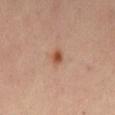workup: total-body-photography surveillance lesion; no biopsy
body site: the abdomen
patient: male, about 65 years old
acquisition: 15 mm crop, total-body photography
lighting: cross-polarized illumination
size: about 2 mm
TBP lesion metrics: a mean CIELAB color near L≈49 a*≈23 b*≈33, about 11 CIELAB-L* units darker than the surrounding skin, and a lesion-to-skin contrast of about 9 (normalized; higher = more distinct); a color-variation rating of about 2/10 and peripheral color asymmetry of about 0.5; a classifier nevus-likeness of about 95/100 and lesion-presence confidence of about 100/100